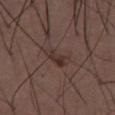This lesion was catalogued during total-body skin photography and was not selected for biopsy. A region of skin cropped from a whole-body photographic capture, roughly 15 mm wide. The subject is a male aged 48–52. Measured at roughly 3.5 mm in maximum diameter. The lesion is located on the abdomen. Captured under white-light illumination. Automated tile analysis of the lesion measured an automated nevus-likeness rating near 30 out of 100.